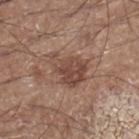biopsy_status: not biopsied; imaged during a skin examination
site: leg
lighting: white-light
image:
  source: total-body photography crop
  field_of_view_mm: 15
patient:
  sex: male
  age_approx: 60
lesion_size:
  long_diameter_mm_approx: 5.0